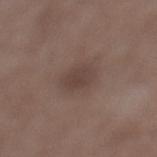Q: Was this lesion biopsied?
A: imaged on a skin check; not biopsied
Q: Automated lesion metrics?
A: a lesion area of about 5.5 mm², a shape eccentricity near 0.7, and a shape-asymmetry score of about 0.15 (0 = symmetric); border irregularity of about 1.5 on a 0–10 scale and internal color variation of about 2 on a 0–10 scale
Q: What lighting was used for the tile?
A: white-light
Q: What kind of image is this?
A: ~15 mm crop, total-body skin-cancer survey
Q: What are the patient's age and sex?
A: male, aged 48–52
Q: Lesion location?
A: the left lower leg
Q: How large is the lesion?
A: ~3 mm (longest diameter)